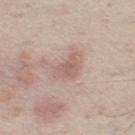Impression:
No biopsy was performed on this lesion — it was imaged during a full skin examination and was not determined to be concerning.
Clinical summary:
The subject is a male aged 63–67. From the leg. Imaged with white-light lighting. A 15 mm close-up tile from a total-body photography series done for melanoma screening.